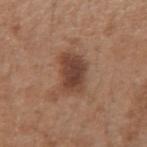Findings:
- biopsy status — catalogued during a skin exam; not biopsied
- diameter — ~4 mm (longest diameter)
- lighting — white-light
- patient — female, roughly 30 years of age
- image — 15 mm crop, total-body photography
- body site — the left forearm